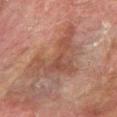follow-up — total-body-photography surveillance lesion; no biopsy | illumination — cross-polarized illumination | site — the arm | subject — male, aged around 75 | lesion diameter — ~7.5 mm (longest diameter) | acquisition — 15 mm crop, total-body photography.